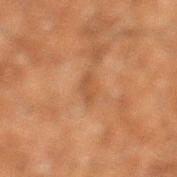Assessment: Captured during whole-body skin photography for melanoma surveillance; the lesion was not biopsied. Clinical summary: A 15 mm crop from a total-body photograph taken for skin-cancer surveillance. Automated tile analysis of the lesion measured a lesion-to-skin contrast of about 5.5 (normalized; higher = more distinct). And it measured a border-irregularity rating of about 3/10 and a within-lesion color-variation index near 0/10. Captured under cross-polarized illumination. A male patient aged approximately 60. The lesion is on the left lower leg.